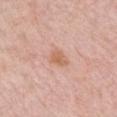biopsy status=total-body-photography surveillance lesion; no biopsy
size=~2.5 mm (longest diameter)
lighting=white-light
patient=female, about 65 years old
image source=15 mm crop, total-body photography
location=the upper back